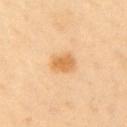Impression: No biopsy was performed on this lesion — it was imaged during a full skin examination and was not determined to be concerning. Image and clinical context: A female subject in their 50s. An algorithmic analysis of the crop reported a classifier nevus-likeness of about 90/100 and a lesion-detection confidence of about 100/100. On the left upper arm. The tile uses cross-polarized illumination. Measured at roughly 3 mm in maximum diameter. A roughly 15 mm field-of-view crop from a total-body skin photograph.